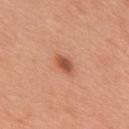<case>
  <site>upper back</site>
  <automated_metrics>
    <eccentricity>0.8</eccentricity>
    <shape_asymmetry>0.15</shape_asymmetry>
    <cielab_L>54</cielab_L>
    <cielab_a>28</cielab_a>
    <cielab_b>34</cielab_b>
    <vs_skin_darker_L>12.0</vs_skin_darker_L>
    <vs_skin_contrast_norm>8.0</vs_skin_contrast_norm>
    <border_irregularity_0_10>1.5</border_irregularity_0_10>
    <color_variation_0_10>3.0</color_variation_0_10>
  </automated_metrics>
  <lighting>white-light</lighting>
  <patient>
    <sex>male</sex>
    <age_approx>70</age_approx>
  </patient>
  <image>
    <source>total-body photography crop</source>
    <field_of_view_mm>15</field_of_view_mm>
  </image>
  <lesion_size>
    <long_diameter_mm_approx>2.5</long_diameter_mm_approx>
  </lesion_size>
</case>